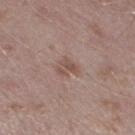Clinical impression:
No biopsy was performed on this lesion — it was imaged during a full skin examination and was not determined to be concerning.
Background:
The total-body-photography lesion software estimated a shape eccentricity near 0.5 and a shape-asymmetry score of about 0.4 (0 = symmetric). It also reported a border-irregularity index near 4/10, a within-lesion color-variation index near 3/10, and radial color variation of about 1. It also reported a nevus-likeness score of about 5/100 and lesion-presence confidence of about 100/100. The lesion is located on the leg. Cropped from a whole-body photographic skin survey; the tile spans about 15 mm. The patient is a male aged approximately 40. This is a white-light tile.